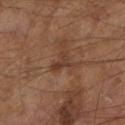follow-up — no biopsy performed (imaged during a skin exam) | lesion diameter — ≈3 mm | lighting — cross-polarized illumination | image — total-body-photography crop, ~15 mm field of view | subject — male, aged 63 to 67 | image-analysis metrics — a lesion color around L≈36 a*≈19 b*≈27 in CIELAB and a lesion–skin lightness drop of about 7; a classifier nevus-likeness of about 0/100 and a lesion-detection confidence of about 100/100.